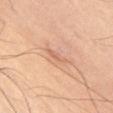Impression:
The lesion was photographed on a routine skin check and not biopsied; there is no pathology result.
Image and clinical context:
The subject is a male in their mid-50s. The lesion is on the abdomen. The tile uses cross-polarized illumination. Longest diameter approximately 3 mm. A roughly 15 mm field-of-view crop from a total-body skin photograph.